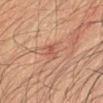Part of a total-body skin-imaging series; this lesion was reviewed on a skin check and was not flagged for biopsy. A male subject aged around 35. Cropped from a whole-body photographic skin survey; the tile spans about 15 mm. Located on the arm. The recorded lesion diameter is about 3 mm. Automated tile analysis of the lesion measured a lesion color around L≈49 a*≈24 b*≈28 in CIELAB, a lesion–skin lightness drop of about 7, and a normalized lesion–skin contrast near 5.5. And it measured a lesion-detection confidence of about 100/100.